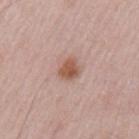follow-up — imaged on a skin check; not biopsied | patient — male, approximately 50 years of age | image — 15 mm crop, total-body photography | body site — the left upper arm.